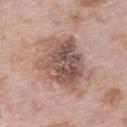acquisition: total-body-photography crop, ~15 mm field of view; body site: the upper back; image-analysis metrics: a classifier nevus-likeness of about 15/100 and a detector confidence of about 100 out of 100 that the crop contains a lesion; subject: female, about 65 years old; lesion diameter: ~7 mm (longest diameter); tile lighting: white-light.